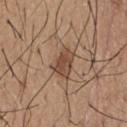Case summary:
- biopsy status · total-body-photography surveillance lesion; no biopsy
- lesion diameter · about 3.5 mm
- TBP lesion metrics · a lesion area of about 7 mm² and an eccentricity of roughly 0.75; a mean CIELAB color near L≈47 a*≈18 b*≈29, a lesion–skin lightness drop of about 11, and a lesion-to-skin contrast of about 8 (normalized; higher = more distinct); border irregularity of about 3.5 on a 0–10 scale, a within-lesion color-variation index near 2.5/10, and a peripheral color-asymmetry measure near 1; a classifier nevus-likeness of about 65/100 and lesion-presence confidence of about 100/100
- body site · the chest
- patient · male, roughly 65 years of age
- acquisition · total-body-photography crop, ~15 mm field of view